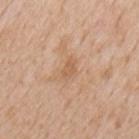The lesion was tiled from a total-body skin photograph and was not biopsied.
Approximately 2.5 mm at its widest.
The lesion is located on the mid back.
This is a white-light tile.
Automated tile analysis of the lesion measured internal color variation of about 1 on a 0–10 scale and peripheral color asymmetry of about 0.5. The software also gave an automated nevus-likeness rating near 0 out of 100 and a detector confidence of about 100 out of 100 that the crop contains a lesion.
A lesion tile, about 15 mm wide, cut from a 3D total-body photograph.
A male patient, aged 63 to 67.